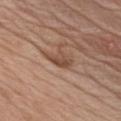biopsy status = catalogued during a skin exam; not biopsied | location = the chest | tile lighting = white-light illumination | image = ~15 mm tile from a whole-body skin photo | size = ≈3.5 mm | patient = male, aged 78–82.